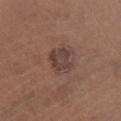Impression: Imaged during a routine full-body skin examination; the lesion was not biopsied and no histopathology is available. Acquisition and patient details: A lesion tile, about 15 mm wide, cut from a 3D total-body photograph. This is a white-light tile. On the right lower leg. The subject is a female approximately 50 years of age.